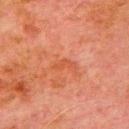Q: Was this lesion biopsied?
A: imaged on a skin check; not biopsied
Q: Illumination type?
A: cross-polarized
Q: Lesion location?
A: the left upper arm
Q: What are the patient's age and sex?
A: male, roughly 80 years of age
Q: What is the imaging modality?
A: ~15 mm crop, total-body skin-cancer survey